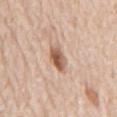This lesion was catalogued during total-body skin photography and was not selected for biopsy.
The lesion-visualizer software estimated an area of roughly 6 mm² and an outline eccentricity of about 0.65 (0 = round, 1 = elongated). The analysis additionally found a lesion color around L≈58 a*≈20 b*≈30 in CIELAB, about 14 CIELAB-L* units darker than the surrounding skin, and a lesion-to-skin contrast of about 9 (normalized; higher = more distinct). The software also gave border irregularity of about 2 on a 0–10 scale and internal color variation of about 6 on a 0–10 scale.
The lesion is located on the back.
The tile uses white-light illumination.
The subject is a male about 80 years old.
A lesion tile, about 15 mm wide, cut from a 3D total-body photograph.
Longest diameter approximately 3 mm.